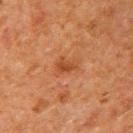| field | value |
|---|---|
| workup | no biopsy performed (imaged during a skin exam) |
| patient | male, aged approximately 60 |
| lesion size | ~3 mm (longest diameter) |
| image source | total-body-photography crop, ~15 mm field of view |
| illumination | cross-polarized |
| site | the right upper arm |